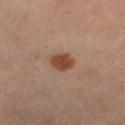| key | value |
|---|---|
| follow-up | total-body-photography surveillance lesion; no biopsy |
| TBP lesion metrics | a footprint of about 5.5 mm², an eccentricity of roughly 0.6, and two-axis asymmetry of about 0.2; border irregularity of about 2 on a 0–10 scale and a within-lesion color-variation index near 2/10; an automated nevus-likeness rating near 100 out of 100 and a detector confidence of about 100 out of 100 that the crop contains a lesion |
| site | the left lower leg |
| illumination | cross-polarized |
| imaging modality | ~15 mm tile from a whole-body skin photo |
| lesion diameter | ~3 mm (longest diameter) |
| subject | female, in their mid-40s |